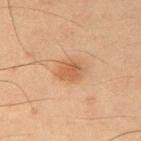biopsy status = imaged on a skin check; not biopsied
site = the right upper arm
image source = ~15 mm tile from a whole-body skin photo
patient = male, approximately 35 years of age
diameter = ≈3 mm
tile lighting = cross-polarized illumination